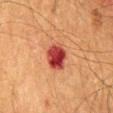Q: Is there a histopathology result?
A: no biopsy performed (imaged during a skin exam)
Q: Where on the body is the lesion?
A: the mid back
Q: How large is the lesion?
A: about 3.5 mm
Q: Illumination type?
A: cross-polarized illumination
Q: What did automated image analysis measure?
A: a classifier nevus-likeness of about 0/100 and a lesion-detection confidence of about 100/100
Q: Patient demographics?
A: male, aged approximately 65
Q: What is the imaging modality?
A: total-body-photography crop, ~15 mm field of view